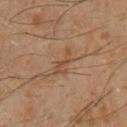Impression: The lesion was tiled from a total-body skin photograph and was not biopsied. Acquisition and patient details: This is a cross-polarized tile. From the chest. The subject is a male about 60 years old. Longest diameter approximately 3.5 mm. A region of skin cropped from a whole-body photographic capture, roughly 15 mm wide.